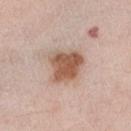Notes:
* workup — catalogued during a skin exam; not biopsied
* imaging modality — ~15 mm tile from a whole-body skin photo
* site — the right upper arm
* subject — male, about 70 years old